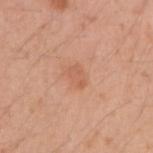The lesion was photographed on a routine skin check and not biopsied; there is no pathology result. On the arm. A roughly 15 mm field-of-view crop from a total-body skin photograph. A male patient aged 28–32.